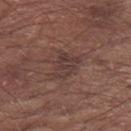{
  "patient": {
    "sex": "male",
    "age_approx": 75
  },
  "site": "left forearm",
  "lesion_size": {
    "long_diameter_mm_approx": 3.5
  },
  "lighting": "white-light",
  "image": {
    "source": "total-body photography crop",
    "field_of_view_mm": 15
  }
}